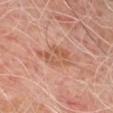notes=catalogued during a skin exam; not biopsied
lighting=cross-polarized illumination
imaging modality=~15 mm tile from a whole-body skin photo
subject=male, roughly 50 years of age
diameter=~5 mm (longest diameter)
site=the chest